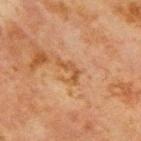Q: Is there a histopathology result?
A: no biopsy performed (imaged during a skin exam)
Q: How was the tile lit?
A: cross-polarized illumination
Q: Patient demographics?
A: male, aged around 65
Q: What did automated image analysis measure?
A: an area of roughly 3 mm², an eccentricity of roughly 0.9, and a shape-asymmetry score of about 0.75 (0 = symmetric); a classifier nevus-likeness of about 0/100 and a lesion-detection confidence of about 100/100
Q: What kind of image is this?
A: 15 mm crop, total-body photography
Q: What is the lesion's diameter?
A: ≈3.5 mm
Q: What is the anatomic site?
A: the chest